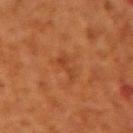A 15 mm close-up extracted from a 3D total-body photography capture. A male patient, roughly 70 years of age. The lesion is located on the right upper arm.